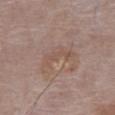This image is a 15 mm lesion crop taken from a total-body photograph. A male patient aged around 85 or older. The lesion is located on the chest.